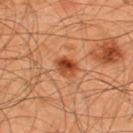The lesion was photographed on a routine skin check and not biopsied; there is no pathology result. The tile uses cross-polarized illumination. Measured at roughly 3 mm in maximum diameter. This image is a 15 mm lesion crop taken from a total-body photograph. A male subject, aged approximately 45. From the upper back. Automated tile analysis of the lesion measured an area of roughly 5.5 mm² and a symmetry-axis asymmetry near 0.2. The software also gave a lesion color around L≈45 a*≈28 b*≈38 in CIELAB and roughly 12 lightness units darker than nearby skin. The software also gave a border-irregularity rating of about 2/10 and peripheral color asymmetry of about 3. The analysis additionally found lesion-presence confidence of about 100/100.